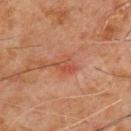No biopsy was performed on this lesion — it was imaged during a full skin examination and was not determined to be concerning.
A male patient, about 60 years old.
The tile uses cross-polarized illumination.
Located on the chest.
The lesion's longest dimension is about 3 mm.
Cropped from a whole-body photographic skin survey; the tile spans about 15 mm.